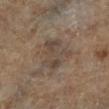Assessment:
Imaged during a routine full-body skin examination; the lesion was not biopsied and no histopathology is available.
Image and clinical context:
Located on the right lower leg. Approximately 4.5 mm at its widest. A male subject in their mid- to late 80s or older. The total-body-photography lesion software estimated an average lesion color of about L≈41 a*≈11 b*≈22 (CIELAB), roughly 7 lightness units darker than nearby skin, and a normalized border contrast of about 6. The software also gave a border-irregularity rating of about 7/10, a color-variation rating of about 3/10, and peripheral color asymmetry of about 1. Cropped from a total-body skin-imaging series; the visible field is about 15 mm.